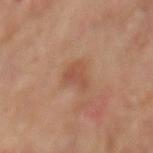Q: Was this lesion biopsied?
A: catalogued during a skin exam; not biopsied
Q: How was the tile lit?
A: cross-polarized
Q: Who is the patient?
A: male, aged 63–67
Q: What did automated image analysis measure?
A: a mean CIELAB color near L≈49 a*≈22 b*≈30, roughly 7 lightness units darker than nearby skin, and a lesion-to-skin contrast of about 5.5 (normalized; higher = more distinct); a border-irregularity rating of about 4/10 and a color-variation rating of about 3/10; an automated nevus-likeness rating near 10 out of 100 and a lesion-detection confidence of about 100/100
Q: What is the imaging modality?
A: total-body-photography crop, ~15 mm field of view
Q: How large is the lesion?
A: about 3.5 mm
Q: What is the anatomic site?
A: the mid back